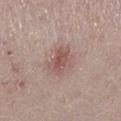Imaged during a routine full-body skin examination; the lesion was not biopsied and no histopathology is available.
This image is a 15 mm lesion crop taken from a total-body photograph.
Measured at roughly 3.5 mm in maximum diameter.
From the left lower leg.
This is a white-light tile.
The patient is a female approximately 20 years of age.